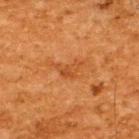Assessment: Imaged during a routine full-body skin examination; the lesion was not biopsied and no histopathology is available. Clinical summary: On the upper back. A male subject roughly 65 years of age. A 15 mm close-up extracted from a 3D total-body photography capture. Longest diameter approximately 3 mm. This is a cross-polarized tile.